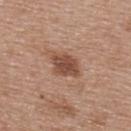notes: imaged on a skin check; not biopsied | automated metrics: an area of roughly 8 mm², an outline eccentricity of about 0.65 (0 = round, 1 = elongated), and a symmetry-axis asymmetry near 0.2; a border-irregularity rating of about 2.5/10, a within-lesion color-variation index near 3/10, and radial color variation of about 1; a classifier nevus-likeness of about 75/100 | anatomic site: the upper back | patient: female, about 40 years old | image: 15 mm crop, total-body photography | tile lighting: white-light illumination | diameter: about 3.5 mm.